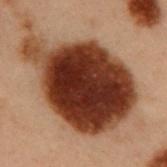{
  "biopsy_status": "not biopsied; imaged during a skin examination",
  "lesion_size": {
    "long_diameter_mm_approx": 12.0
  },
  "automated_metrics": {
    "area_mm2_approx": 65.0,
    "shape_asymmetry": 0.3
  },
  "patient": {
    "sex": "male",
    "age_approx": 55
  },
  "lighting": "cross-polarized",
  "image": {
    "source": "total-body photography crop",
    "field_of_view_mm": 15
  },
  "site": "arm"
}